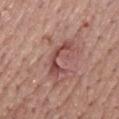Impression: The lesion was tiled from a total-body skin photograph and was not biopsied. Background: Automated tile analysis of the lesion measured a footprint of about 6 mm², an eccentricity of roughly 0.9, and a symmetry-axis asymmetry near 0.75. It also reported a lesion color around L≈47 a*≈25 b*≈24 in CIELAB, a lesion–skin lightness drop of about 11, and a lesion-to-skin contrast of about 8 (normalized; higher = more distinct). The software also gave an automated nevus-likeness rating near 0 out of 100 and a lesion-detection confidence of about 60/100. A roughly 15 mm field-of-view crop from a total-body skin photograph. The lesion is on the mid back. Measured at roughly 4.5 mm in maximum diameter. The tile uses white-light illumination. The patient is a male aged 73–77.